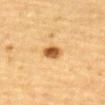Case summary:
• follow-up: catalogued during a skin exam; not biopsied
• image source: ~15 mm tile from a whole-body skin photo
• location: the abdomen
• tile lighting: cross-polarized illumination
• patient: male, aged approximately 85 or older
• size: ~2.5 mm (longest diameter)